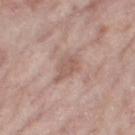Q: Is there a histopathology result?
A: no biopsy performed (imaged during a skin exam)
Q: Illumination type?
A: white-light illumination
Q: Automated lesion metrics?
A: about 8 CIELAB-L* units darker than the surrounding skin and a normalized lesion–skin contrast near 6; lesion-presence confidence of about 100/100
Q: How large is the lesion?
A: ≈4 mm
Q: Who is the patient?
A: female, aged 68–72
Q: Where on the body is the lesion?
A: the right thigh
Q: How was this image acquired?
A: ~15 mm crop, total-body skin-cancer survey